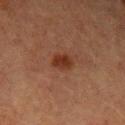Assessment:
The lesion was photographed on a routine skin check and not biopsied; there is no pathology result.
Image and clinical context:
On the left upper arm. A 15 mm crop from a total-body photograph taken for skin-cancer surveillance. Automated tile analysis of the lesion measured a shape eccentricity near 0.6. And it measured an average lesion color of about L≈27 a*≈21 b*≈26 (CIELAB), roughly 8 lightness units darker than nearby skin, and a lesion-to-skin contrast of about 8.5 (normalized; higher = more distinct). The software also gave a border-irregularity rating of about 2/10 and a peripheral color-asymmetry measure near 1. It also reported a nevus-likeness score of about 90/100 and a detector confidence of about 100 out of 100 that the crop contains a lesion. Captured under cross-polarized illumination. The recorded lesion diameter is about 2.5 mm. The patient is a female aged around 55.